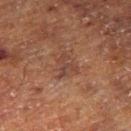lighting = cross-polarized illumination | anatomic site = the right thigh | image source = 15 mm crop, total-body photography | subject = male, aged approximately 75.